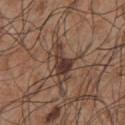- workup — total-body-photography surveillance lesion; no biopsy
- image — ~15 mm crop, total-body skin-cancer survey
- diameter — ≈3.5 mm
- body site — the front of the torso
- tile lighting — white-light illumination
- subject — male, about 55 years old